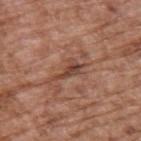Q: Was a biopsy performed?
A: no biopsy performed (imaged during a skin exam)
Q: Lesion size?
A: ≈4 mm
Q: What is the anatomic site?
A: the upper back
Q: Who is the patient?
A: male, in their mid-70s
Q: What is the imaging modality?
A: total-body-photography crop, ~15 mm field of view